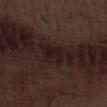Q: Is there a histopathology result?
A: no biopsy performed (imaged during a skin exam)
Q: Illumination type?
A: white-light illumination
Q: Lesion size?
A: ~3 mm (longest diameter)
Q: What did automated image analysis measure?
A: an average lesion color of about L≈16 a*≈15 b*≈13 (CIELAB), a lesion–skin lightness drop of about 6, and a lesion-to-skin contrast of about 8 (normalized; higher = more distinct); a color-variation rating of about 1/10 and peripheral color asymmetry of about 0; a classifier nevus-likeness of about 0/100 and a detector confidence of about 90 out of 100 that the crop contains a lesion
Q: What is the imaging modality?
A: ~15 mm tile from a whole-body skin photo
Q: What are the patient's age and sex?
A: male, roughly 70 years of age
Q: Where on the body is the lesion?
A: the leg